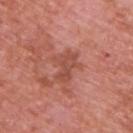Q: Is there a histopathology result?
A: imaged on a skin check; not biopsied
Q: Illumination type?
A: white-light
Q: What are the patient's age and sex?
A: male, roughly 70 years of age
Q: What did automated image analysis measure?
A: an average lesion color of about L≈50 a*≈28 b*≈29 (CIELAB) and roughly 8 lightness units darker than nearby skin; a border-irregularity rating of about 6/10 and a color-variation rating of about 2.5/10
Q: What is the imaging modality?
A: total-body-photography crop, ~15 mm field of view
Q: What is the anatomic site?
A: the upper back